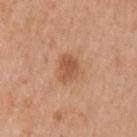Imaged during a routine full-body skin examination; the lesion was not biopsied and no histopathology is available. A 15 mm crop from a total-body photograph taken for skin-cancer surveillance. The subject is a female in their mid- to late 50s. On the left upper arm. About 3 mm across.